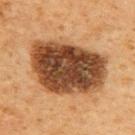{
  "biopsy_status": "not biopsied; imaged during a skin examination",
  "image": {
    "source": "total-body photography crop",
    "field_of_view_mm": 15
  },
  "patient": {
    "sex": "male",
    "age_approx": 60
  },
  "lighting": "cross-polarized",
  "site": "upper back",
  "lesion_size": {
    "long_diameter_mm_approx": 9.0
  }
}